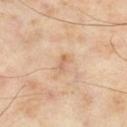A male subject, in their mid- to late 50s.
Automated image analysis of the tile measured an area of roughly 2.5 mm² and a shape-asymmetry score of about 0.3 (0 = symmetric). The software also gave an average lesion color of about L≈65 a*≈19 b*≈34 (CIELAB), a lesion–skin lightness drop of about 8, and a normalized lesion–skin contrast near 5.5. The software also gave a border-irregularity rating of about 3/10 and a peripheral color-asymmetry measure near 0. It also reported an automated nevus-likeness rating near 5 out of 100 and a lesion-detection confidence of about 100/100.
Located on the left thigh.
A close-up tile cropped from a whole-body skin photograph, about 15 mm across.
The lesion's longest dimension is about 2.5 mm.
This is a cross-polarized tile.